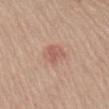Q: Was a biopsy performed?
A: imaged on a skin check; not biopsied
Q: Lesion size?
A: about 3.5 mm
Q: Patient demographics?
A: male, aged around 65
Q: Automated lesion metrics?
A: a mean CIELAB color near L≈58 a*≈22 b*≈27, roughly 8 lightness units darker than nearby skin, and a normalized lesion–skin contrast near 5.5; a classifier nevus-likeness of about 30/100 and a detector confidence of about 100 out of 100 that the crop contains a lesion
Q: How was this image acquired?
A: ~15 mm crop, total-body skin-cancer survey
Q: Where on the body is the lesion?
A: the upper back